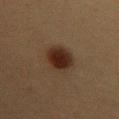{
  "biopsy_status": "not biopsied; imaged during a skin examination",
  "image": {
    "source": "total-body photography crop",
    "field_of_view_mm": 15
  },
  "patient": {
    "sex": "female",
    "age_approx": 40
  },
  "site": "chest",
  "automated_metrics": {
    "cielab_L": 23,
    "cielab_a": 16,
    "cielab_b": 22,
    "vs_skin_darker_L": 11.0,
    "vs_skin_contrast_norm": 12.0,
    "border_irregularity_0_10": 1.5,
    "color_variation_0_10": 3.5,
    "peripheral_color_asymmetry": 1.0
  },
  "lighting": "cross-polarized",
  "lesion_size": {
    "long_diameter_mm_approx": 4.0
  }
}